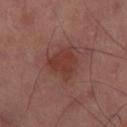follow-up: total-body-photography surveillance lesion; no biopsy | tile lighting: cross-polarized | imaging modality: total-body-photography crop, ~15 mm field of view | size: ≈3.5 mm | anatomic site: the right thigh.